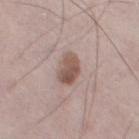  biopsy_status: not biopsied; imaged during a skin examination
  image:
    source: total-body photography crop
    field_of_view_mm: 15
  site: left thigh
  patient:
    sex: male
    age_approx: 65
  lesion_size:
    long_diameter_mm_approx: 4.0
  lighting: white-light
  automated_metrics:
    cielab_L: 53
    cielab_a: 17
    cielab_b: 23
    vs_skin_darker_L: 12.0
    vs_skin_contrast_norm: 8.5
    border_irregularity_0_10: 2.0
    color_variation_0_10: 5.0
    peripheral_color_asymmetry: 2.0
    nevus_likeness_0_100: 45
    lesion_detection_confidence_0_100: 100